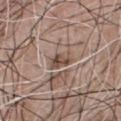<record>
<biopsy_status>not biopsied; imaged during a skin examination</biopsy_status>
<lighting>white-light</lighting>
<automated_metrics>
  <shape_asymmetry>0.25</shape_asymmetry>
  <nevus_likeness_0_100>0</nevus_likeness_0_100>
  <lesion_detection_confidence_0_100>55</lesion_detection_confidence_0_100>
</automated_metrics>
<patient>
  <sex>male</sex>
  <age_approx>75</age_approx>
</patient>
<image>
  <source>total-body photography crop</source>
  <field_of_view_mm>15</field_of_view_mm>
</image>
<lesion_size>
  <long_diameter_mm_approx>3.0</long_diameter_mm_approx>
</lesion_size>
<site>chest</site>
</record>